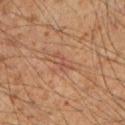Imaged during a routine full-body skin examination; the lesion was not biopsied and no histopathology is available.
On the right upper arm.
The lesion-visualizer software estimated an average lesion color of about L≈46 a*≈21 b*≈28 (CIELAB) and a normalized lesion–skin contrast near 5. The analysis additionally found a border-irregularity index near 7.5/10, a color-variation rating of about 0/10, and a peripheral color-asymmetry measure near 0.
A male subject aged approximately 50.
Longest diameter approximately 3 mm.
A lesion tile, about 15 mm wide, cut from a 3D total-body photograph.
The tile uses cross-polarized illumination.